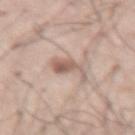This lesion was catalogued during total-body skin photography and was not selected for biopsy. A 15 mm crop from a total-body photograph taken for skin-cancer surveillance. Automated tile analysis of the lesion measured an area of roughly 5.5 mm², a shape eccentricity near 0.8, and a symmetry-axis asymmetry near 0.5. It also reported a within-lesion color-variation index near 4/10 and radial color variation of about 1.5. It also reported an automated nevus-likeness rating near 65 out of 100. The lesion's longest dimension is about 4 mm. A male subject, aged 38–42. From the right thigh.